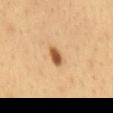biopsy_status: not biopsied; imaged during a skin examination
lighting: cross-polarized
patient:
  sex: male
  age_approx: 35
lesion_size:
  long_diameter_mm_approx: 2.5
site: mid back
automated_metrics:
  shape_asymmetry: 0.2
  vs_skin_contrast_norm: 10.5
  border_irregularity_0_10: 2.0
  color_variation_0_10: 3.5
  peripheral_color_asymmetry: 1.0
image:
  source: total-body photography crop
  field_of_view_mm: 15